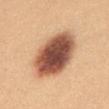Q: Is there a histopathology result?
A: catalogued during a skin exam; not biopsied
Q: Lesion location?
A: the chest
Q: Patient demographics?
A: female, in their mid- to late 20s
Q: What kind of image is this?
A: ~15 mm crop, total-body skin-cancer survey